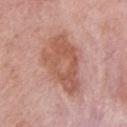Part of a total-body skin-imaging series; this lesion was reviewed on a skin check and was not flagged for biopsy. A female subject, in their mid-60s. The lesion is on the right upper arm. A region of skin cropped from a whole-body photographic capture, roughly 15 mm wide.